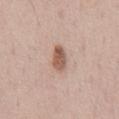Clinical impression: Recorded during total-body skin imaging; not selected for excision or biopsy. Acquisition and patient details: From the chest. The patient is a male aged 68–72. A 15 mm crop from a total-body photograph taken for skin-cancer surveillance.